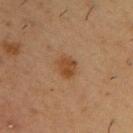A male patient, aged 53 to 57. Located on the arm. A lesion tile, about 15 mm wide, cut from a 3D total-body photograph.